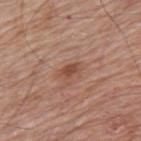Context: Longest diameter approximately 2.5 mm. A lesion tile, about 15 mm wide, cut from a 3D total-body photograph. The tile uses white-light illumination. A male subject aged around 80. On the mid back.